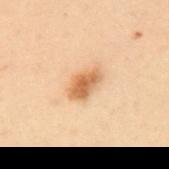The lesion is located on the upper back.
The patient is a female approximately 50 years of age.
Captured under cross-polarized illumination.
A roughly 15 mm field-of-view crop from a total-body skin photograph.
About 4 mm across.